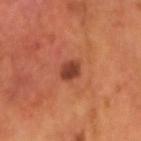<tbp_lesion>
  <biopsy_status>not biopsied; imaged during a skin examination</biopsy_status>
  <image>
    <source>total-body photography crop</source>
    <field_of_view_mm>15</field_of_view_mm>
  </image>
  <patient>
    <sex>male</sex>
    <age_approx>55</age_approx>
  </patient>
  <site>head or neck</site>
</tbp_lesion>